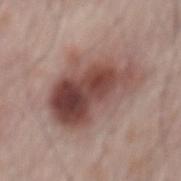workup = catalogued during a skin exam; not biopsied | tile lighting = white-light illumination | lesion diameter = about 8 mm | imaging modality = ~15 mm tile from a whole-body skin photo | site = the mid back | subject = male, roughly 65 years of age.